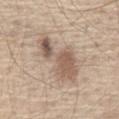Clinical impression: The lesion was tiled from a total-body skin photograph and was not biopsied. Clinical summary: A male subject aged approximately 65. Approximately 7 mm at its widest. The lesion is on the abdomen. This image is a 15 mm lesion crop taken from a total-body photograph.